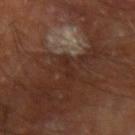Measured at roughly 2.5 mm in maximum diameter.
The patient is a male roughly 65 years of age.
The total-body-photography lesion software estimated a lesion area of about 2 mm². The software also gave border irregularity of about 5.5 on a 0–10 scale, internal color variation of about 0 on a 0–10 scale, and a peripheral color-asymmetry measure near 0.
The lesion is located on the right forearm.
A 15 mm close-up tile from a total-body photography series done for melanoma screening.
Imaged with cross-polarized lighting.